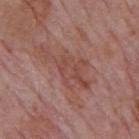{
  "biopsy_status": "not biopsied; imaged during a skin examination",
  "image": {
    "source": "total-body photography crop",
    "field_of_view_mm": 15
  },
  "site": "mid back",
  "patient": {
    "sex": "male",
    "age_approx": 75
  },
  "lesion_size": {
    "long_diameter_mm_approx": 7.0
  }
}